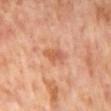<tbp_lesion>
<biopsy_status>not biopsied; imaged during a skin examination</biopsy_status>
<lesion_size>
  <long_diameter_mm_approx>3.5</long_diameter_mm_approx>
</lesion_size>
<patient>
  <sex>male</sex>
  <age_approx>65</age_approx>
</patient>
<lighting>cross-polarized</lighting>
<image>
  <source>total-body photography crop</source>
  <field_of_view_mm>15</field_of_view_mm>
</image>
<site>mid back</site>
<automated_metrics>
  <eccentricity>0.85</eccentricity>
  <shape_asymmetry>0.25</shape_asymmetry>
  <border_irregularity_0_10>2.5</border_irregularity_0_10>
  <peripheral_color_asymmetry>2.0</peripheral_color_asymmetry>
</automated_metrics>
</tbp_lesion>